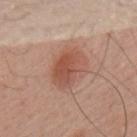This lesion was catalogued during total-body skin photography and was not selected for biopsy. The patient is a male about 60 years old. Automated image analysis of the tile measured an average lesion color of about L≈53 a*≈23 b*≈29 (CIELAB) and about 10 CIELAB-L* units darker than the surrounding skin. Longest diameter approximately 5 mm. The lesion is located on the upper back. Imaged with white-light lighting. A 15 mm close-up tile from a total-body photography series done for melanoma screening.